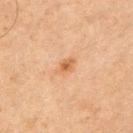– workup — catalogued during a skin exam; not biopsied
– illumination — cross-polarized
– image — ~15 mm crop, total-body skin-cancer survey
– diameter — ~2.5 mm (longest diameter)
– body site — the left upper arm
– patient — male, in their mid- to late 60s
– image-analysis metrics — a lesion area of about 3 mm²; an average lesion color of about L≈51 a*≈20 b*≈35 (CIELAB), about 8 CIELAB-L* units darker than the surrounding skin, and a normalized lesion–skin contrast near 7; border irregularity of about 2.5 on a 0–10 scale, a color-variation rating of about 2.5/10, and peripheral color asymmetry of about 0.5; an automated nevus-likeness rating near 50 out of 100 and a detector confidence of about 100 out of 100 that the crop contains a lesion